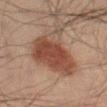Impression: Captured during whole-body skin photography for melanoma surveillance; the lesion was not biopsied. Background: The patient is a male aged 68–72. The lesion is on the left thigh. A 15 mm crop from a total-body photograph taken for skin-cancer surveillance.